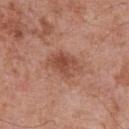Context:
A male patient in their 70s. A close-up tile cropped from a whole-body skin photograph, about 15 mm across. The lesion is on the chest. The lesion's longest dimension is about 4 mm.